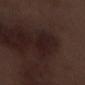workup = imaged on a skin check; not biopsied | lighting = white-light | body site = the right lower leg | patient = male, aged approximately 70 | diameter = about 2.5 mm | image = total-body-photography crop, ~15 mm field of view.